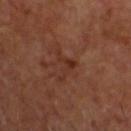follow-up: total-body-photography surveillance lesion; no biopsy | body site: the head or neck | subject: male, approximately 60 years of age | tile lighting: cross-polarized | image source: total-body-photography crop, ~15 mm field of view.